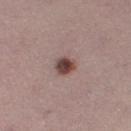Findings:
– workup · imaged on a skin check; not biopsied
– lesion diameter · ≈3 mm
– imaging modality · ~15 mm crop, total-body skin-cancer survey
– subject · female, aged 23 to 27
– site · the right thigh
– image-analysis metrics · roughly 15 lightness units darker than nearby skin and a normalized lesion–skin contrast near 11